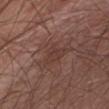Assessment: Recorded during total-body skin imaging; not selected for excision or biopsy. Background: The total-body-photography lesion software estimated a footprint of about 4.5 mm², an outline eccentricity of about 0.75 (0 = round, 1 = elongated), and a shape-asymmetry score of about 0.45 (0 = symmetric). And it measured about 5 CIELAB-L* units darker than the surrounding skin. And it measured a border-irregularity rating of about 4/10 and a peripheral color-asymmetry measure near 0.5. It also reported a lesion-detection confidence of about 85/100. The tile uses white-light illumination. Approximately 3.5 mm at its widest. Located on the abdomen. The subject is a male aged approximately 65. A lesion tile, about 15 mm wide, cut from a 3D total-body photograph.